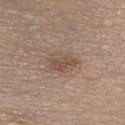{"biopsy_status": "not biopsied; imaged during a skin examination", "image": {"source": "total-body photography crop", "field_of_view_mm": 15}, "lesion_size": {"long_diameter_mm_approx": 3.5}, "patient": {"sex": "female", "age_approx": 65}, "site": "chest"}